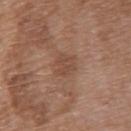Q: Is there a histopathology result?
A: no biopsy performed (imaged during a skin exam)
Q: What are the patient's age and sex?
A: female, aged 68–72
Q: Lesion size?
A: ≈2.5 mm
Q: What lighting was used for the tile?
A: white-light
Q: Automated lesion metrics?
A: a lesion color around L≈47 a*≈20 b*≈28 in CIELAB, about 7 CIELAB-L* units darker than the surrounding skin, and a normalized border contrast of about 5
Q: What is the anatomic site?
A: the upper back
Q: What kind of image is this?
A: total-body-photography crop, ~15 mm field of view